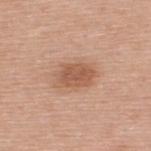Acquisition and patient details:
This image is a 15 mm lesion crop taken from a total-body photograph. This is a white-light tile. The lesion's longest dimension is about 4.5 mm. A male subject, aged around 45. From the back.